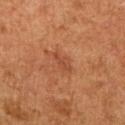Q: Was this lesion biopsied?
A: imaged on a skin check; not biopsied
Q: What are the patient's age and sex?
A: female, roughly 60 years of age
Q: Lesion location?
A: the left upper arm
Q: How was this image acquired?
A: 15 mm crop, total-body photography